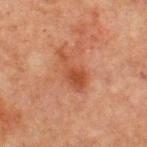biopsy_status: not biopsied; imaged during a skin examination
image:
  source: total-body photography crop
  field_of_view_mm: 15
lighting: cross-polarized
lesion_size:
  long_diameter_mm_approx: 5.0
site: chest
patient:
  sex: male
  age_approx: 65